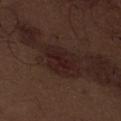Impression:
This lesion was catalogued during total-body skin photography and was not selected for biopsy.
Acquisition and patient details:
The lesion is on the front of the torso. Imaged with white-light lighting. The total-body-photography lesion software estimated an area of roughly 10 mm², a shape eccentricity near 0.75, and a symmetry-axis asymmetry near 0.25. It also reported a lesion color around L≈20 a*≈17 b*≈17 in CIELAB and a normalized border contrast of about 8.5. The software also gave border irregularity of about 3.5 on a 0–10 scale, a color-variation rating of about 2.5/10, and a peripheral color-asymmetry measure near 1. The software also gave a classifier nevus-likeness of about 35/100 and a lesion-detection confidence of about 95/100. A region of skin cropped from a whole-body photographic capture, roughly 15 mm wide. A male patient about 70 years old.